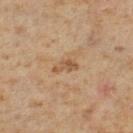workup = total-body-photography surveillance lesion; no biopsy.